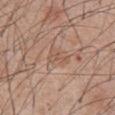follow-up = catalogued during a skin exam; not biopsied | illumination = white-light illumination | anatomic site = the abdomen | image source = ~15 mm tile from a whole-body skin photo | subject = male, aged 58 to 62.